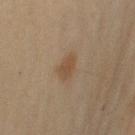Captured during whole-body skin photography for melanoma surveillance; the lesion was not biopsied.
The patient is a female roughly 60 years of age.
On the right upper arm.
An algorithmic analysis of the crop reported a lesion area of about 4 mm², an eccentricity of roughly 0.8, and a shape-asymmetry score of about 0.25 (0 = symmetric). The software also gave a within-lesion color-variation index near 1.5/10 and a peripheral color-asymmetry measure near 0.5.
Approximately 3 mm at its widest.
A close-up tile cropped from a whole-body skin photograph, about 15 mm across.
This is a cross-polarized tile.